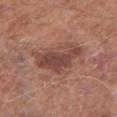Recorded during total-body skin imaging; not selected for excision or biopsy. A male subject aged around 65. A close-up tile cropped from a whole-body skin photograph, about 15 mm across. Located on the right thigh. Captured under white-light illumination.